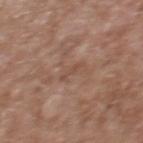Q: Was this lesion biopsied?
A: no biopsy performed (imaged during a skin exam)
Q: What kind of image is this?
A: total-body-photography crop, ~15 mm field of view
Q: Lesion size?
A: ≈2.5 mm
Q: Illumination type?
A: white-light
Q: What did automated image analysis measure?
A: an outline eccentricity of about 0.95 (0 = round, 1 = elongated) and a shape-asymmetry score of about 0.4 (0 = symmetric); a mean CIELAB color near L≈48 a*≈19 b*≈26 and a normalized border contrast of about 5
Q: Patient demographics?
A: male, aged approximately 45
Q: Lesion location?
A: the upper back